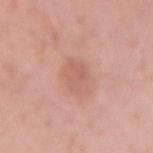The lesion was photographed on a routine skin check and not biopsied; there is no pathology result. Cropped from a whole-body photographic skin survey; the tile spans about 15 mm. The lesion is located on the right upper arm. The subject is a male aged 43 to 47.